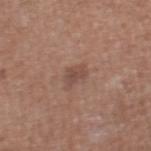Clinical impression:
Recorded during total-body skin imaging; not selected for excision or biopsy.